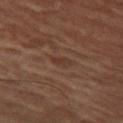Imaged during a routine full-body skin examination; the lesion was not biopsied and no histopathology is available.
From the left thigh.
A male subject approximately 60 years of age.
A 15 mm close-up extracted from a 3D total-body photography capture.
About 2.5 mm across.